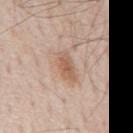A region of skin cropped from a whole-body photographic capture, roughly 15 mm wide. From the back. The tile uses white-light illumination. A male patient roughly 80 years of age. Measured at roughly 4 mm in maximum diameter. The lesion-visualizer software estimated an average lesion color of about L≈59 a*≈19 b*≈30 (CIELAB), a lesion–skin lightness drop of about 10, and a normalized border contrast of about 7.